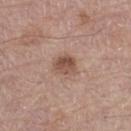| key | value |
|---|---|
| workup | catalogued during a skin exam; not biopsied |
| subject | male, aged approximately 75 |
| acquisition | ~15 mm crop, total-body skin-cancer survey |
| location | the left thigh |